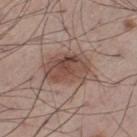A 15 mm crop from a total-body photograph taken for skin-cancer surveillance. The total-body-photography lesion software estimated an area of roughly 16 mm². The analysis additionally found roughly 11 lightness units darker than nearby skin and a lesion-to-skin contrast of about 8 (normalized; higher = more distinct). It also reported a border-irregularity index near 2.5/10 and a color-variation rating of about 6/10. It also reported a lesion-detection confidence of about 100/100. A male subject roughly 60 years of age. The lesion is located on the left thigh. Imaged with white-light lighting. The recorded lesion diameter is about 5.5 mm.